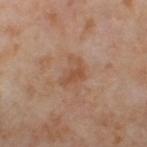The lesion was tiled from a total-body skin photograph and was not biopsied. The lesion's longest dimension is about 3.5 mm. On the leg. A roughly 15 mm field-of-view crop from a total-body skin photograph. A female patient, approximately 55 years of age. Automated image analysis of the tile measured an area of roughly 5 mm², an outline eccentricity of about 0.75 (0 = round, 1 = elongated), and a shape-asymmetry score of about 0.55 (0 = symmetric). It also reported a mean CIELAB color near L≈51 a*≈20 b*≈32. The software also gave a border-irregularity rating of about 6/10.